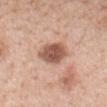Acquisition and patient details:
This is a white-light tile. A female patient, roughly 50 years of age. A 15 mm close-up tile from a total-body photography series done for melanoma screening. The total-body-photography lesion software estimated a classifier nevus-likeness of about 65/100 and lesion-presence confidence of about 100/100. Located on the mid back.